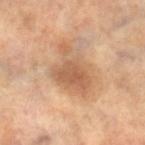Findings:
* biopsy status — no biopsy performed (imaged during a skin exam)
* image-analysis metrics — a shape eccentricity near 0.75 and two-axis asymmetry of about 0.45; a lesion color around L≈57 a*≈20 b*≈34 in CIELAB and a lesion-to-skin contrast of about 7 (normalized; higher = more distinct); a border-irregularity rating of about 5.5/10, a within-lesion color-variation index near 3/10, and peripheral color asymmetry of about 1
* anatomic site — the left leg
* imaging modality — total-body-photography crop, ~15 mm field of view
* subject — female, roughly 65 years of age
* lesion diameter — ~6 mm (longest diameter)